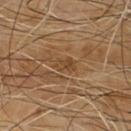biopsy status: imaged on a skin check; not biopsied
patient: male, aged 63 to 67
imaging modality: ~15 mm tile from a whole-body skin photo